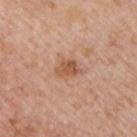Clinical impression:
The lesion was photographed on a routine skin check and not biopsied; there is no pathology result.
Background:
Captured under white-light illumination. The subject is a male approximately 75 years of age. The recorded lesion diameter is about 3 mm. This image is a 15 mm lesion crop taken from a total-body photograph. On the left upper arm.